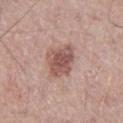Findings:
- anatomic site · the leg
- lesion diameter · about 4 mm
- image · 15 mm crop, total-body photography
- automated metrics · a footprint of about 11 mm², an outline eccentricity of about 0.45 (0 = round, 1 = elongated), and two-axis asymmetry of about 0.25; a lesion color around L≈53 a*≈20 b*≈23 in CIELAB and a normalized border contrast of about 8; internal color variation of about 4 on a 0–10 scale and peripheral color asymmetry of about 1; an automated nevus-likeness rating near 55 out of 100
- subject · male, aged around 75